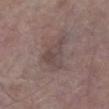Clinical impression:
No biopsy was performed on this lesion — it was imaged during a full skin examination and was not determined to be concerning.
Image and clinical context:
Longest diameter approximately 4.5 mm. Imaged with white-light lighting. This image is a 15 mm lesion crop taken from a total-body photograph. The lesion is located on the left lower leg. A male subject, approximately 80 years of age. Automated tile analysis of the lesion measured a lesion area of about 9.5 mm². The software also gave an average lesion color of about L≈45 a*≈14 b*≈17 (CIELAB), about 7 CIELAB-L* units darker than the surrounding skin, and a lesion-to-skin contrast of about 5.5 (normalized; higher = more distinct). The analysis additionally found border irregularity of about 5 on a 0–10 scale, internal color variation of about 3.5 on a 0–10 scale, and radial color variation of about 1.5.